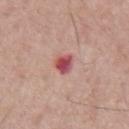An algorithmic analysis of the crop reported an area of roughly 4 mm², a shape eccentricity near 0.7, and two-axis asymmetry of about 0.25. The analysis additionally found a border-irregularity index near 2/10 and a color-variation rating of about 5.5/10. It also reported an automated nevus-likeness rating near 0 out of 100 and a detector confidence of about 100 out of 100 that the crop contains a lesion.
The patient is a male aged 63 to 67.
Cropped from a total-body skin-imaging series; the visible field is about 15 mm.
On the mid back.